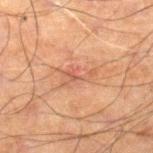Clinical impression:
The lesion was photographed on a routine skin check and not biopsied; there is no pathology result.
Context:
The lesion is located on the leg. A 15 mm crop from a total-body photograph taken for skin-cancer surveillance. An algorithmic analysis of the crop reported a footprint of about 6.5 mm², a shape eccentricity near 0.85, and two-axis asymmetry of about 0.45. The analysis additionally found a color-variation rating of about 4.5/10 and a peripheral color-asymmetry measure near 1.5. Imaged with cross-polarized lighting. The patient is a male about 60 years old.